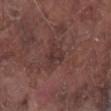Captured during whole-body skin photography for melanoma surveillance; the lesion was not biopsied. The subject is a male in their mid- to late 70s. The recorded lesion diameter is about 2.5 mm. From the right lower leg. A 15 mm crop from a total-body photograph taken for skin-cancer surveillance.